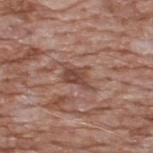Assessment: No biopsy was performed on this lesion — it was imaged during a full skin examination and was not determined to be concerning.